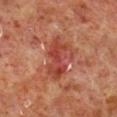Notes:
• biopsy status · no biopsy performed (imaged during a skin exam)
• size · ≈5 mm
• image source · ~15 mm crop, total-body skin-cancer survey
• site · the right lower leg
• tile lighting · cross-polarized illumination
• subject · male, about 60 years old